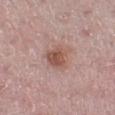Q: What is the lesion's diameter?
A: about 3.5 mm
Q: How was this image acquired?
A: 15 mm crop, total-body photography
Q: Where on the body is the lesion?
A: the leg
Q: What are the patient's age and sex?
A: female, aged around 45